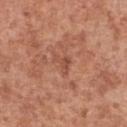Impression: Captured during whole-body skin photography for melanoma surveillance; the lesion was not biopsied.